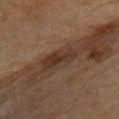image: 15 mm crop, total-body photography | lesion diameter: ≈5 mm | lighting: cross-polarized illumination | site: the upper back | patient: male, about 85 years old or older.